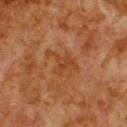follow-up — imaged on a skin check; not biopsied
imaging modality — total-body-photography crop, ~15 mm field of view
subject — male, aged around 80
lesion size — ~4 mm (longest diameter)
lighting — cross-polarized illumination
image-analysis metrics — a nevus-likeness score of about 0/100 and a lesion-detection confidence of about 100/100
anatomic site — the back